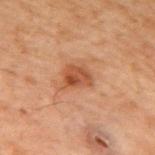| field | value |
|---|---|
| image source | 15 mm crop, total-body photography |
| automated metrics | an area of roughly 6 mm² and a symmetry-axis asymmetry near 0.3; a lesion color around L≈38 a*≈21 b*≈29 in CIELAB and roughly 9 lightness units darker than nearby skin; a border-irregularity rating of about 2.5/10, a color-variation rating of about 4.5/10, and radial color variation of about 1.5 |
| body site | the upper back |
| diameter | about 3 mm |
| lighting | cross-polarized illumination |
| patient | male, aged 68 to 72 |